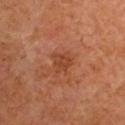biopsy status: catalogued during a skin exam; not biopsied
image source: 15 mm crop, total-body photography
patient: male, about 65 years old
tile lighting: cross-polarized
diameter: ≈2.5 mm
automated lesion analysis: a footprint of about 5 mm², an eccentricity of roughly 0.4, and a shape-asymmetry score of about 0.3 (0 = symmetric); border irregularity of about 3.5 on a 0–10 scale and peripheral color asymmetry of about 1
site: the arm